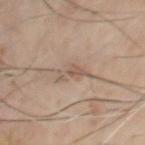Imaged during a routine full-body skin examination; the lesion was not biopsied and no histopathology is available.
A male patient in their mid- to late 60s.
A roughly 15 mm field-of-view crop from a total-body skin photograph.
Imaged with cross-polarized lighting.
The recorded lesion diameter is about 4 mm.
From the chest.